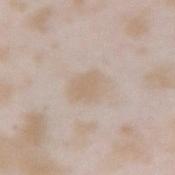This lesion was catalogued during total-body skin photography and was not selected for biopsy. A 15 mm close-up extracted from a 3D total-body photography capture. The recorded lesion diameter is about 4 mm. Captured under white-light illumination. An algorithmic analysis of the crop reported a lesion area of about 8.5 mm², an eccentricity of roughly 0.65, and a symmetry-axis asymmetry near 0.2. And it measured a nevus-likeness score of about 10/100. A female patient aged 23–27. On the right forearm.